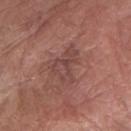The lesion was tiled from a total-body skin photograph and was not biopsied. Located on the right forearm. The lesion's longest dimension is about 4.5 mm. A 15 mm close-up extracted from a 3D total-body photography capture. A female subject, in their mid- to late 70s. Captured under white-light illumination.